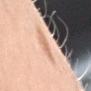Clinical impression: Captured during whole-body skin photography for melanoma surveillance; the lesion was not biopsied. Clinical summary: Captured under white-light illumination. A roughly 15 mm field-of-view crop from a total-body skin photograph. Located on the left upper arm. Measured at roughly 2.5 mm in maximum diameter. A female patient aged around 40. The lesion-visualizer software estimated a lesion–skin lightness drop of about 8 and a normalized border contrast of about 5. The software also gave border irregularity of about 3.5 on a 0–10 scale and a peripheral color-asymmetry measure near 0.5.